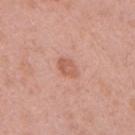  biopsy_status: not biopsied; imaged during a skin examination
  image:
    source: total-body photography crop
    field_of_view_mm: 15
  patient:
    sex: female
    age_approx: 40
  site: right upper arm
  lighting: white-light